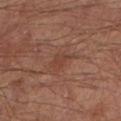{
  "lesion_size": {
    "long_diameter_mm_approx": 3.0
  },
  "lighting": "cross-polarized",
  "image": {
    "source": "total-body photography crop",
    "field_of_view_mm": 15
  },
  "site": "left lower leg",
  "patient": {
    "sex": "male",
    "age_approx": 65
  }
}